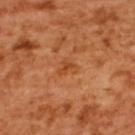Impression:
Part of a total-body skin-imaging series; this lesion was reviewed on a skin check and was not flagged for biopsy.
Clinical summary:
A lesion tile, about 15 mm wide, cut from a 3D total-body photograph. A female patient, aged 53 to 57. This is a cross-polarized tile. The recorded lesion diameter is about 2.5 mm. The lesion is located on the upper back.